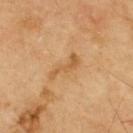follow-up — imaged on a skin check; not biopsied
location — the upper back
imaging modality — ~15 mm tile from a whole-body skin photo
patient — male, about 70 years old
tile lighting — cross-polarized